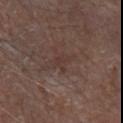{
  "biopsy_status": "not biopsied; imaged during a skin examination",
  "site": "left forearm",
  "image": {
    "source": "total-body photography crop",
    "field_of_view_mm": 15
  },
  "patient": {
    "sex": "male",
    "age_approx": 80
  },
  "lighting": "white-light",
  "automated_metrics": {
    "cielab_L": 36,
    "cielab_a": 16,
    "cielab_b": 21,
    "vs_skin_darker_L": 5.0,
    "vs_skin_contrast_norm": 5.0,
    "peripheral_color_asymmetry": 0.5,
    "nevus_likeness_0_100": 0,
    "lesion_detection_confidence_0_100": 85
  }
}